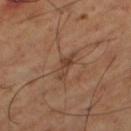biopsy status = imaged on a skin check; not biopsied
location = the leg
patient = male, aged 63–67
imaging modality = 15 mm crop, total-body photography